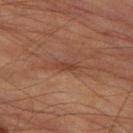Q: Is there a histopathology result?
A: imaged on a skin check; not biopsied
Q: Patient demographics?
A: male, in their mid-80s or older
Q: What lighting was used for the tile?
A: cross-polarized
Q: What is the anatomic site?
A: the left thigh
Q: What is the imaging modality?
A: 15 mm crop, total-body photography
Q: How large is the lesion?
A: about 2.5 mm